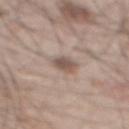The lesion was tiled from a total-body skin photograph and was not biopsied. A region of skin cropped from a whole-body photographic capture, roughly 15 mm wide. A male subject, in their 50s. The tile uses white-light illumination. On the mid back. The total-body-photography lesion software estimated a lesion area of about 4.5 mm² and a shape-asymmetry score of about 0.15 (0 = symmetric). And it measured a lesion color around L≈53 a*≈15 b*≈23 in CIELAB, roughly 12 lightness units darker than nearby skin, and a lesion-to-skin contrast of about 8 (normalized; higher = more distinct). The software also gave a border-irregularity rating of about 1.5/10, a color-variation rating of about 3/10, and peripheral color asymmetry of about 1. The recorded lesion diameter is about 2.5 mm.